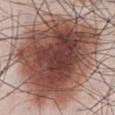Notes:
- biopsy status · no biopsy performed (imaged during a skin exam)
- lesion size · about 12.5 mm
- tile lighting · white-light illumination
- anatomic site · the abdomen
- imaging modality · ~15 mm tile from a whole-body skin photo
- patient · male, aged approximately 35
- automated metrics · border irregularity of about 2.5 on a 0–10 scale, a within-lesion color-variation index near 8.5/10, and radial color variation of about 2.5